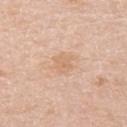Q: Is there a histopathology result?
A: total-body-photography surveillance lesion; no biopsy
Q: How was this image acquired?
A: total-body-photography crop, ~15 mm field of view
Q: Automated lesion metrics?
A: border irregularity of about 3 on a 0–10 scale, a within-lesion color-variation index near 1.5/10, and peripheral color asymmetry of about 0.5; a nevus-likeness score of about 0/100 and lesion-presence confidence of about 100/100
Q: How large is the lesion?
A: ~2.5 mm (longest diameter)
Q: What is the anatomic site?
A: the left upper arm
Q: What are the patient's age and sex?
A: female, roughly 45 years of age